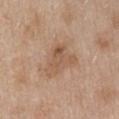biopsy_status: not biopsied; imaged during a skin examination
site: front of the torso
image:
  source: total-body photography crop
  field_of_view_mm: 15
patient:
  sex: female
  age_approx: 40
lesion_size:
  long_diameter_mm_approx: 4.5